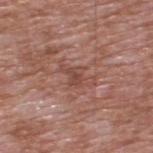Clinical summary: From the upper back. The recorded lesion diameter is about 4 mm. This is a white-light tile. The lesion-visualizer software estimated an area of roughly 5.5 mm², a shape eccentricity near 0.8, and a symmetry-axis asymmetry near 0.45. The analysis additionally found a lesion color around L≈47 a*≈23 b*≈25 in CIELAB and a lesion-to-skin contrast of about 5.5 (normalized; higher = more distinct). The software also gave border irregularity of about 6 on a 0–10 scale and peripheral color asymmetry of about 0.5. The software also gave a detector confidence of about 80 out of 100 that the crop contains a lesion. A male subject, in their 60s. A roughly 15 mm field-of-view crop from a total-body skin photograph.